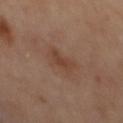The lesion was tiled from a total-body skin photograph and was not biopsied.